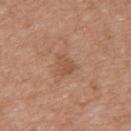  biopsy_status: not biopsied; imaged during a skin examination
  patient:
    sex: female
    age_approx: 70
  site: upper back
  image:
    source: total-body photography crop
    field_of_view_mm: 15